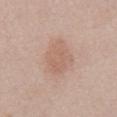workup — catalogued during a skin exam; not biopsied | body site — the abdomen | acquisition — ~15 mm crop, total-body skin-cancer survey | subject — male, approximately 55 years of age | diameter — ≈4.5 mm.